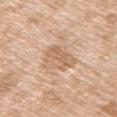{
  "biopsy_status": "not biopsied; imaged during a skin examination",
  "image": {
    "source": "total-body photography crop",
    "field_of_view_mm": 15
  },
  "site": "arm",
  "patient": {
    "sex": "male",
    "age_approx": 65
  }
}